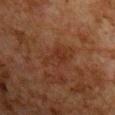Clinical impression:
Part of a total-body skin-imaging series; this lesion was reviewed on a skin check and was not flagged for biopsy.
Context:
Longest diameter approximately 4 mm. A lesion tile, about 15 mm wide, cut from a 3D total-body photograph. A male subject aged 78–82. The lesion is on the right upper arm. The lesion-visualizer software estimated a footprint of about 5.5 mm², an eccentricity of roughly 0.85, and a shape-asymmetry score of about 0.4 (0 = symmetric). And it measured about 4 CIELAB-L* units darker than the surrounding skin and a lesion-to-skin contrast of about 5.5 (normalized; higher = more distinct). Captured under cross-polarized illumination.